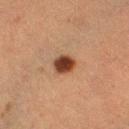Imaged during a routine full-body skin examination; the lesion was not biopsied and no histopathology is available. A female subject, aged approximately 55. The recorded lesion diameter is about 2.5 mm. Captured under cross-polarized illumination. The total-body-photography lesion software estimated about 15 CIELAB-L* units darker than the surrounding skin and a normalized border contrast of about 12.5. A 15 mm close-up extracted from a 3D total-body photography capture. The lesion is located on the right thigh.